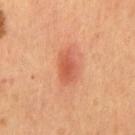Q: Is there a histopathology result?
A: imaged on a skin check; not biopsied
Q: What is the anatomic site?
A: the chest
Q: Who is the patient?
A: male, approximately 55 years of age
Q: What is the lesion's diameter?
A: ~4 mm (longest diameter)
Q: What kind of image is this?
A: ~15 mm crop, total-body skin-cancer survey
Q: What lighting was used for the tile?
A: cross-polarized